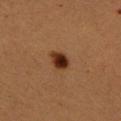A female subject aged around 55. Imaged with cross-polarized lighting. Measured at roughly 2.5 mm in maximum diameter. Cropped from a whole-body photographic skin survey; the tile spans about 15 mm. The lesion is on the left forearm.